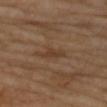Captured during whole-body skin photography for melanoma surveillance; the lesion was not biopsied. The recorded lesion diameter is about 2.5 mm. Captured under cross-polarized illumination. A roughly 15 mm field-of-view crop from a total-body skin photograph. On the right upper arm. A female patient, in their 70s. Automated image analysis of the tile measured an automated nevus-likeness rating near 0 out of 100 and lesion-presence confidence of about 100/100.